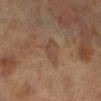Notes:
– subject — female, aged 63–67
– anatomic site — the left leg
– acquisition — total-body-photography crop, ~15 mm field of view